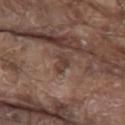The lesion was tiled from a total-body skin photograph and was not biopsied.